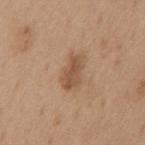Part of a total-body skin-imaging series; this lesion was reviewed on a skin check and was not flagged for biopsy. Longest diameter approximately 4 mm. A region of skin cropped from a whole-body photographic capture, roughly 15 mm wide. A male patient, in their mid- to late 60s. Imaged with white-light lighting. The lesion is located on the chest. The total-body-photography lesion software estimated a border-irregularity index near 3.5/10, a within-lesion color-variation index near 2.5/10, and peripheral color asymmetry of about 1. The analysis additionally found a classifier nevus-likeness of about 40/100 and a detector confidence of about 100 out of 100 that the crop contains a lesion.